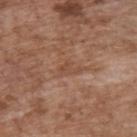patient: male, aged approximately 70 | lighting: white-light | diameter: about 2.5 mm | image source: ~15 mm crop, total-body skin-cancer survey | body site: the upper back.